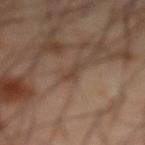{"biopsy_status": "not biopsied; imaged during a skin examination", "lesion_size": {"long_diameter_mm_approx": 3.0}, "image": {"source": "total-body photography crop", "field_of_view_mm": 15}, "patient": {"sex": "male", "age_approx": 65}, "site": "abdomen"}